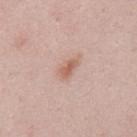Recorded during total-body skin imaging; not selected for excision or biopsy.
From the mid back.
The total-body-photography lesion software estimated an area of roughly 3 mm² and two-axis asymmetry of about 0.25. And it measured an average lesion color of about L≈60 a*≈22 b*≈28 (CIELAB).
Approximately 2.5 mm at its widest.
Captured under white-light illumination.
A male patient, roughly 25 years of age.
A 15 mm crop from a total-body photograph taken for skin-cancer surveillance.